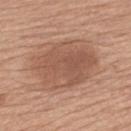From the upper back. Imaged with white-light lighting. The subject is a female approximately 65 years of age. Cropped from a whole-body photographic skin survey; the tile spans about 15 mm. Automated tile analysis of the lesion measured a mean CIELAB color near L≈54 a*≈21 b*≈29, about 9 CIELAB-L* units darker than the surrounding skin, and a normalized lesion–skin contrast near 6.5. The analysis additionally found a lesion-detection confidence of about 100/100. Longest diameter approximately 8 mm.